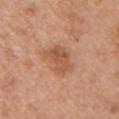Part of a total-body skin-imaging series; this lesion was reviewed on a skin check and was not flagged for biopsy. Cropped from a whole-body photographic skin survey; the tile spans about 15 mm. An algorithmic analysis of the crop reported an average lesion color of about L≈54 a*≈24 b*≈34 (CIELAB), a lesion–skin lightness drop of about 9, and a lesion-to-skin contrast of about 6.5 (normalized; higher = more distinct). The analysis additionally found a classifier nevus-likeness of about 25/100 and a detector confidence of about 100 out of 100 that the crop contains a lesion. The lesion's longest dimension is about 4 mm. The subject is a female about 40 years old. From the left upper arm.